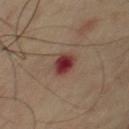This lesion was catalogued during total-body skin photography and was not selected for biopsy.
The lesion's longest dimension is about 3 mm.
This image is a 15 mm lesion crop taken from a total-body photograph.
The lesion is located on the chest.
The total-body-photography lesion software estimated a shape eccentricity near 0.75 and a shape-asymmetry score of about 0.1 (0 = symmetric). It also reported a lesion-detection confidence of about 100/100.
A male patient, aged approximately 70.
This is a cross-polarized tile.